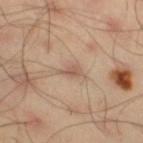{
  "biopsy_status": "not biopsied; imaged during a skin examination",
  "automated_metrics": {
    "eccentricity": 0.85,
    "cielab_L": 55,
    "cielab_a": 18,
    "cielab_b": 27,
    "vs_skin_darker_L": 8.0,
    "vs_skin_contrast_norm": 6.0,
    "nevus_likeness_0_100": 0,
    "lesion_detection_confidence_0_100": 100
  },
  "site": "right thigh",
  "lesion_size": {
    "long_diameter_mm_approx": 3.0
  },
  "lighting": "cross-polarized",
  "patient": {
    "sex": "male",
    "age_approx": 45
  },
  "image": {
    "source": "total-body photography crop",
    "field_of_view_mm": 15
  }
}